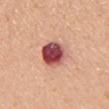biopsy status=catalogued during a skin exam; not biopsied | imaging modality=~15 mm tile from a whole-body skin photo | patient=female, approximately 40 years of age | site=the front of the torso.